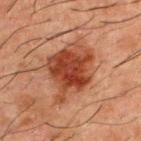Captured during whole-body skin photography for melanoma surveillance; the lesion was not biopsied. Automated image analysis of the tile measured a within-lesion color-variation index near 5.5/10. The software also gave a nevus-likeness score of about 90/100 and a lesion-detection confidence of about 100/100. On the back. Cropped from a whole-body photographic skin survey; the tile spans about 15 mm. A male subject aged 48–52. Measured at roughly 6.5 mm in maximum diameter. The tile uses cross-polarized illumination.